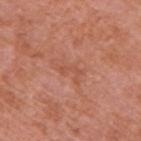Imaged during a routine full-body skin examination; the lesion was not biopsied and no histopathology is available. This is a white-light tile. The total-body-photography lesion software estimated a footprint of about 2 mm² and a shape eccentricity near 0.95. It also reported an average lesion color of about L≈53 a*≈27 b*≈33 (CIELAB) and a lesion-to-skin contrast of about 4.5 (normalized; higher = more distinct). And it measured a border-irregularity rating of about 6/10, a within-lesion color-variation index near 0/10, and a peripheral color-asymmetry measure near 0. Located on the right upper arm. A male patient approximately 70 years of age. Measured at roughly 2.5 mm in maximum diameter. A region of skin cropped from a whole-body photographic capture, roughly 15 mm wide.